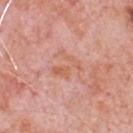Cropped from a total-body skin-imaging series; the visible field is about 15 mm. The lesion is located on the chest. A male subject, approximately 80 years of age. Measured at roughly 3.5 mm in maximum diameter.